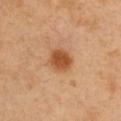notes: no biopsy performed (imaged during a skin exam) | location: the chest | lesion size: about 3 mm | image source: 15 mm crop, total-body photography | tile lighting: cross-polarized illumination | subject: male, in their 50s.